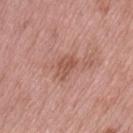Assessment:
Imaged during a routine full-body skin examination; the lesion was not biopsied and no histopathology is available.
Background:
The patient is a female in their 70s. The lesion is on the leg. The lesion's longest dimension is about 3 mm. A roughly 15 mm field-of-view crop from a total-body skin photograph.